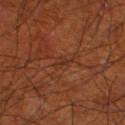automated metrics = a lesion color around L≈31 a*≈24 b*≈29 in CIELAB and a normalized border contrast of about 5.5; a within-lesion color-variation index near 1/10 and a peripheral color-asymmetry measure near 0.5; a classifier nevus-likeness of about 0/100 and a lesion-detection confidence of about 70/100
size = about 2.5 mm
image source = ~15 mm tile from a whole-body skin photo
location = the left lower leg
patient = male, roughly 70 years of age